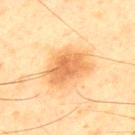<lesion>
  <biopsy_status>not biopsied; imaged during a skin examination</biopsy_status>
  <lighting>cross-polarized</lighting>
  <automated_metrics>
    <border_irregularity_0_10>2.5</border_irregularity_0_10>
    <color_variation_0_10>3.0</color_variation_0_10>
    <peripheral_color_asymmetry>1.0</peripheral_color_asymmetry>
  </automated_metrics>
  <lesion_size>
    <long_diameter_mm_approx>5.0</long_diameter_mm_approx>
  </lesion_size>
  <image>
    <source>total-body photography crop</source>
    <field_of_view_mm>15</field_of_view_mm>
  </image>
  <patient>
    <sex>male</sex>
    <age_approx>45</age_approx>
  </patient>
  <site>back</site>
</lesion>